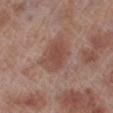Assessment:
The lesion was tiled from a total-body skin photograph and was not biopsied.
Background:
From the left lower leg. The lesion-visualizer software estimated a lesion color around L≈47 a*≈22 b*≈25 in CIELAB, about 8 CIELAB-L* units darker than the surrounding skin, and a lesion-to-skin contrast of about 6.5 (normalized; higher = more distinct). Cropped from a total-body skin-imaging series; the visible field is about 15 mm. The lesion's longest dimension is about 4 mm. Captured under white-light illumination. The subject is a male aged 68–72.